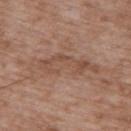No biopsy was performed on this lesion — it was imaged during a full skin examination and was not determined to be concerning. The lesion is on the upper back. Cropped from a whole-body photographic skin survey; the tile spans about 15 mm. This is a white-light tile. Automated image analysis of the tile measured an area of roughly 14 mm² and a shape-asymmetry score of about 0.35 (0 = symmetric). And it measured a lesion color around L≈50 a*≈19 b*≈28 in CIELAB and a lesion-to-skin contrast of about 5.5 (normalized; higher = more distinct). And it measured an automated nevus-likeness rating near 0 out of 100 and a detector confidence of about 90 out of 100 that the crop contains a lesion. A male patient roughly 50 years of age.